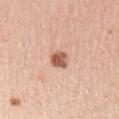Assessment: The lesion was tiled from a total-body skin photograph and was not biopsied. Background: A close-up tile cropped from a whole-body skin photograph, about 15 mm across. Automated image analysis of the tile measured a lesion color around L≈58 a*≈23 b*≈30 in CIELAB. And it measured a within-lesion color-variation index near 3.5/10 and a peripheral color-asymmetry measure near 1. And it measured lesion-presence confidence of about 100/100. The lesion is on the left upper arm. A female subject in their mid-40s. About 2.5 mm across.